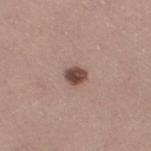Imaged during a routine full-body skin examination; the lesion was not biopsied and no histopathology is available. A region of skin cropped from a whole-body photographic capture, roughly 15 mm wide. The subject is a female aged 43 to 47. The recorded lesion diameter is about 2.5 mm. Captured under white-light illumination. The lesion is on the right thigh. Automated tile analysis of the lesion measured a lesion area of about 4 mm², an outline eccentricity of about 0.5 (0 = round, 1 = elongated), and a shape-asymmetry score of about 0.2 (0 = symmetric).